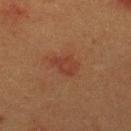workup: no biopsy performed (imaged during a skin exam)
illumination: cross-polarized illumination
imaging modality: 15 mm crop, total-body photography
lesion size: ≈3.5 mm
anatomic site: the upper back
image-analysis metrics: an area of roughly 5 mm² and two-axis asymmetry of about 0.3; an average lesion color of about L≈32 a*≈22 b*≈26 (CIELAB); border irregularity of about 3 on a 0–10 scale, a color-variation rating of about 1.5/10, and peripheral color asymmetry of about 0.5
patient: male, about 40 years old